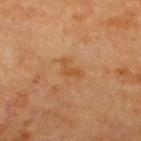Context:
The tile uses cross-polarized illumination. The subject is about 65 years old. About 3 mm across. The lesion is located on the upper back. A 15 mm close-up tile from a total-body photography series done for melanoma screening.